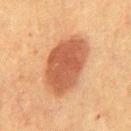<record>
  <biopsy_status>not biopsied; imaged during a skin examination</biopsy_status>
  <site>chest</site>
  <patient>
    <sex>male</sex>
    <age_approx>50</age_approx>
  </patient>
  <lesion_size>
    <long_diameter_mm_approx>7.5</long_diameter_mm_approx>
  </lesion_size>
  <lighting>cross-polarized</lighting>
  <image>
    <source>total-body photography crop</source>
    <field_of_view_mm>15</field_of_view_mm>
  </image>
</record>